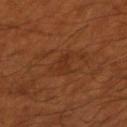subject: male, aged around 55 | body site: the right forearm | imaging modality: 15 mm crop, total-body photography | lighting: cross-polarized illumination | lesion diameter: ~2.5 mm (longest diameter).